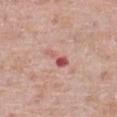Assessment: Part of a total-body skin-imaging series; this lesion was reviewed on a skin check and was not flagged for biopsy. Acquisition and patient details: A 15 mm close-up extracted from a 3D total-body photography capture. This is a white-light tile. The patient is a male aged around 80. From the front of the torso.